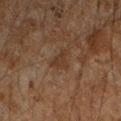Clinical impression:
This lesion was catalogued during total-body skin photography and was not selected for biopsy.
Context:
Approximately 2.5 mm at its widest. Imaged with cross-polarized lighting. Cropped from a total-body skin-imaging series; the visible field is about 15 mm. Automated tile analysis of the lesion measured a border-irregularity rating of about 4.5/10, internal color variation of about 1 on a 0–10 scale, and peripheral color asymmetry of about 0.5. It also reported a lesion-detection confidence of about 100/100. A male subject about 45 years old. The lesion is on the left forearm.